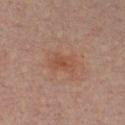No biopsy was performed on this lesion — it was imaged during a full skin examination and was not determined to be concerning.